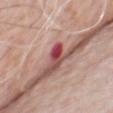Clinical impression: The lesion was photographed on a routine skin check and not biopsied; there is no pathology result. Context: Imaged with white-light lighting. The lesion is on the chest. The subject is a male aged 78 to 82. A close-up tile cropped from a whole-body skin photograph, about 15 mm across. The recorded lesion diameter is about 3.5 mm. The total-body-photography lesion software estimated two-axis asymmetry of about 0.3. And it measured an average lesion color of about L≈46 a*≈33 b*≈23 (CIELAB), a lesion–skin lightness drop of about 18, and a lesion-to-skin contrast of about 12.5 (normalized; higher = more distinct). And it measured border irregularity of about 3.5 on a 0–10 scale and a within-lesion color-variation index near 7/10. It also reported a classifier nevus-likeness of about 0/100 and a lesion-detection confidence of about 100/100.